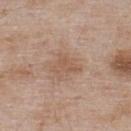biopsy status=imaged on a skin check; not biopsied | image-analysis metrics=a lesion area of about 6 mm² and a shape eccentricity near 0.55; roughly 7 lightness units darker than nearby skin and a normalized lesion–skin contrast near 5.5; border irregularity of about 6 on a 0–10 scale, internal color variation of about 2 on a 0–10 scale, and peripheral color asymmetry of about 1; a classifier nevus-likeness of about 0/100 and a detector confidence of about 100 out of 100 that the crop contains a lesion | body site=the upper back | image=15 mm crop, total-body photography | patient=male, aged approximately 55 | lesion size=≈3.5 mm.